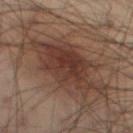<case>
  <biopsy_status>not biopsied; imaged during a skin examination</biopsy_status>
  <site>left thigh</site>
  <automated_metrics>
    <area_mm2_approx>27.0</area_mm2_approx>
    <eccentricity>0.9</eccentricity>
    <shape_asymmetry>0.25</shape_asymmetry>
    <cielab_L>29</cielab_L>
    <cielab_a>15</cielab_a>
    <cielab_b>20</cielab_b>
    <vs_skin_darker_L>8.0</vs_skin_darker_L>
    <vs_skin_contrast_norm>8.5</vs_skin_contrast_norm>
    <border_irregularity_0_10>5.5</border_irregularity_0_10>
    <color_variation_0_10>3.5</color_variation_0_10>
    <peripheral_color_asymmetry>1.0</peripheral_color_asymmetry>
    <lesion_detection_confidence_0_100>100</lesion_detection_confidence_0_100>
  </automated_metrics>
  <patient>
    <sex>male</sex>
    <age_approx>55</age_approx>
  </patient>
  <image>
    <source>total-body photography crop</source>
    <field_of_view_mm>15</field_of_view_mm>
  </image>
  <lighting>cross-polarized</lighting>
</case>